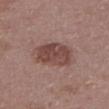Clinical impression:
Part of a total-body skin-imaging series; this lesion was reviewed on a skin check and was not flagged for biopsy.
Context:
Longest diameter approximately 5 mm. A female subject about 65 years old. On the upper back. This is a white-light tile. A 15 mm close-up tile from a total-body photography series done for melanoma screening.